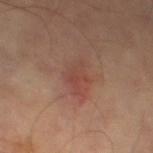biopsy status: imaged on a skin check; not biopsied | site: the leg | imaging modality: ~15 mm crop, total-body skin-cancer survey.